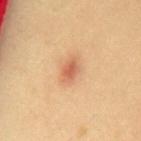{
  "biopsy_status": "not biopsied; imaged during a skin examination",
  "automated_metrics": {
    "eccentricity": 0.8,
    "shape_asymmetry": 0.25
  },
  "site": "mid back",
  "image": {
    "source": "total-body photography crop",
    "field_of_view_mm": 15
  },
  "patient": {
    "sex": "female",
    "age_approx": 35
  },
  "lighting": "cross-polarized"
}